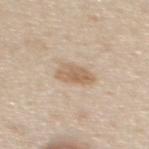Part of a total-body skin-imaging series; this lesion was reviewed on a skin check and was not flagged for biopsy. A close-up tile cropped from a whole-body skin photograph, about 15 mm across. A female patient approximately 45 years of age. This is a white-light tile. Automated tile analysis of the lesion measured a border-irregularity rating of about 2.5/10 and peripheral color asymmetry of about 1. It also reported a classifier nevus-likeness of about 70/100 and a detector confidence of about 100 out of 100 that the crop contains a lesion. Longest diameter approximately 4 mm. Located on the upper back.